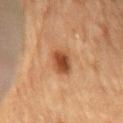Impression: The lesion was photographed on a routine skin check and not biopsied; there is no pathology result. Context: Longest diameter approximately 3 mm. Cropped from a whole-body photographic skin survey; the tile spans about 15 mm. A male subject aged approximately 85 or older. The lesion is located on the back. Automated image analysis of the tile measured a lesion color around L≈41 a*≈22 b*≈33 in CIELAB, roughly 13 lightness units darker than nearby skin, and a normalized lesion–skin contrast near 10. The software also gave a border-irregularity rating of about 1.5/10, internal color variation of about 3.5 on a 0–10 scale, and radial color variation of about 1. This is a cross-polarized tile.